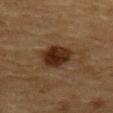This is a cross-polarized tile. A male patient in their mid-80s or older. From the back. Longest diameter approximately 3.5 mm. A 15 mm crop from a total-body photograph taken for skin-cancer surveillance.